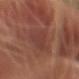The lesion-visualizer software estimated a lesion area of about 11 mm² and an outline eccentricity of about 0.9 (0 = round, 1 = elongated). And it measured a mean CIELAB color near L≈40 a*≈25 b*≈25 and a normalized border contrast of about 5.
Imaged with white-light lighting.
Located on the head or neck.
A female subject aged approximately 80.
The lesion's longest dimension is about 5.5 mm.
A lesion tile, about 15 mm wide, cut from a 3D total-body photograph.